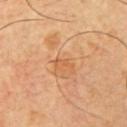Clinical impression:
Imaged during a routine full-body skin examination; the lesion was not biopsied and no histopathology is available.
Context:
A male subject aged approximately 65. A 15 mm close-up tile from a total-body photography series done for melanoma screening. Approximately 2.5 mm at its widest. The tile uses cross-polarized illumination. An algorithmic analysis of the crop reported a border-irregularity index near 5/10, a within-lesion color-variation index near 0/10, and a peripheral color-asymmetry measure near 0. The analysis additionally found lesion-presence confidence of about 100/100. The lesion is on the left upper arm.